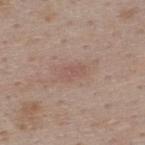Q: Is there a histopathology result?
A: total-body-photography surveillance lesion; no biopsy
Q: Automated lesion metrics?
A: a shape eccentricity near 0.85 and a shape-asymmetry score of about 0.3 (0 = symmetric)
Q: Patient demographics?
A: female, in their mid-40s
Q: What kind of image is this?
A: ~15 mm crop, total-body skin-cancer survey
Q: What is the lesion's diameter?
A: about 3 mm
Q: What is the anatomic site?
A: the upper back